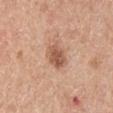follow-up: total-body-photography surveillance lesion; no biopsy
imaging modality: total-body-photography crop, ~15 mm field of view
anatomic site: the right upper arm
automated lesion analysis: a footprint of about 7 mm² and a shape eccentricity near 0.7; a mean CIELAB color near L≈56 a*≈22 b*≈31, roughly 11 lightness units darker than nearby skin, and a normalized border contrast of about 7.5; a border-irregularity rating of about 2/10, internal color variation of about 3.5 on a 0–10 scale, and radial color variation of about 1
subject: male, in their 70s
diameter: ~3.5 mm (longest diameter)
tile lighting: white-light illumination